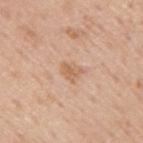Recorded during total-body skin imaging; not selected for excision or biopsy. A 15 mm crop from a total-body photograph taken for skin-cancer surveillance. A male patient aged 73–77. This is a white-light tile. Located on the right upper arm. About 2.5 mm across.